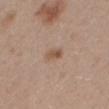A roughly 15 mm field-of-view crop from a total-body skin photograph.
A female patient, aged around 40.
This is a white-light tile.
Longest diameter approximately 2.5 mm.
On the mid back.